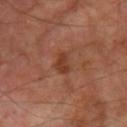Impression:
This lesion was catalogued during total-body skin photography and was not selected for biopsy.
Context:
A male subject, about 70 years old. Imaged with cross-polarized lighting. Cropped from a total-body skin-imaging series; the visible field is about 15 mm. About 2.5 mm across. Located on the right thigh.